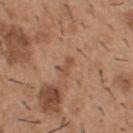Recorded during total-body skin imaging; not selected for excision or biopsy. A male patient, aged 38 to 42. A 15 mm crop from a total-body photograph taken for skin-cancer surveillance. On the back.